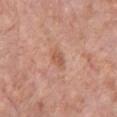Q: Is there a histopathology result?
A: imaged on a skin check; not biopsied
Q: What are the patient's age and sex?
A: male, aged around 80
Q: What is the imaging modality?
A: ~15 mm crop, total-body skin-cancer survey
Q: Where on the body is the lesion?
A: the chest